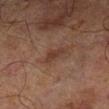From the right lower leg. A region of skin cropped from a whole-body photographic capture, roughly 15 mm wide. A male subject aged around 70. Automated tile analysis of the lesion measured an area of roughly 3 mm², an outline eccentricity of about 0.85 (0 = round, 1 = elongated), and two-axis asymmetry of about 0.3. The software also gave a detector confidence of about 100 out of 100 that the crop contains a lesion. Captured under cross-polarized illumination.